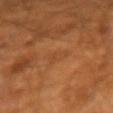• biopsy status: total-body-photography surveillance lesion; no biopsy
• tile lighting: cross-polarized illumination
• subject: male, about 50 years old
• location: the right forearm
• acquisition: ~15 mm tile from a whole-body skin photo
• TBP lesion metrics: a mean CIELAB color near L≈44 a*≈23 b*≈37 and a lesion-to-skin contrast of about 3 (normalized; higher = more distinct); border irregularity of about 3 on a 0–10 scale, internal color variation of about 1.5 on a 0–10 scale, and peripheral color asymmetry of about 0.5